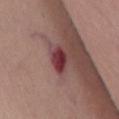Clinical impression:
No biopsy was performed on this lesion — it was imaged during a full skin examination and was not determined to be concerning.
Background:
A roughly 15 mm field-of-view crop from a total-body skin photograph. On the chest. Longest diameter approximately 3 mm. A female subject aged approximately 65. Captured under white-light illumination.